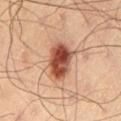The lesion was tiled from a total-body skin photograph and was not biopsied.